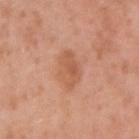Case summary:
– workup · imaged on a skin check; not biopsied
– site · the right upper arm
– patient · female, aged approximately 40
– lesion diameter · ≈4 mm
– tile lighting · white-light
– TBP lesion metrics · a lesion area of about 8 mm², an outline eccentricity of about 0.7 (0 = round, 1 = elongated), and a shape-asymmetry score of about 0.2 (0 = symmetric); a border-irregularity rating of about 2/10
– acquisition · 15 mm crop, total-body photography